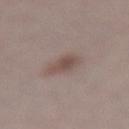<tbp_lesion>
  <biopsy_status>not biopsied; imaged during a skin examination</biopsy_status>
  <lighting>white-light</lighting>
  <lesion_size>
    <long_diameter_mm_approx>3.5</long_diameter_mm_approx>
  </lesion_size>
  <image>
    <source>total-body photography crop</source>
    <field_of_view_mm>15</field_of_view_mm>
  </image>
  <site>lower back</site>
  <patient>
    <sex>female</sex>
    <age_approx>50</age_approx>
  </patient>
</tbp_lesion>